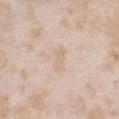Assessment:
Part of a total-body skin-imaging series; this lesion was reviewed on a skin check and was not flagged for biopsy.
Image and clinical context:
A region of skin cropped from a whole-body photographic capture, roughly 15 mm wide. The patient is a female about 25 years old. From the chest.